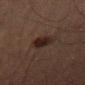biopsy_status: not biopsied; imaged during a skin examination
site: left thigh
automated_metrics:
  area_mm2_approx: 6.0
  eccentricity: 0.65
  cielab_L: 17
  cielab_a: 12
  cielab_b: 16
  vs_skin_darker_L: 7.0
  vs_skin_contrast_norm: 10.0
  border_irregularity_0_10: 2.0
  peripheral_color_asymmetry: 1.0
  lesion_detection_confidence_0_100: 100
patient:
  sex: male
  age_approx: 65
image:
  source: total-body photography crop
  field_of_view_mm: 15
lesion_size:
  long_diameter_mm_approx: 3.0